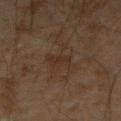Clinical impression: The lesion was photographed on a routine skin check and not biopsied; there is no pathology result. Image and clinical context: An algorithmic analysis of the crop reported a lesion-detection confidence of about 95/100. The tile uses cross-polarized illumination. The lesion is located on the left forearm. Measured at roughly 2.5 mm in maximum diameter. This image is a 15 mm lesion crop taken from a total-body photograph. The patient is a male approximately 45 years of age.